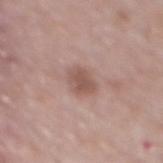The lesion was photographed on a routine skin check and not biopsied; there is no pathology result. A male patient, approximately 70 years of age. A region of skin cropped from a whole-body photographic capture, roughly 15 mm wide. This is a white-light tile. Measured at roughly 3 mm in maximum diameter. The lesion is located on the back. Automated tile analysis of the lesion measured about 10 CIELAB-L* units darker than the surrounding skin and a normalized border contrast of about 7. And it measured border irregularity of about 2 on a 0–10 scale, a within-lesion color-variation index near 2/10, and radial color variation of about 0.5. The software also gave lesion-presence confidence of about 100/100.